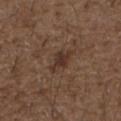<tbp_lesion>
  <biopsy_status>not biopsied; imaged during a skin examination</biopsy_status>
  <site>arm</site>
  <image>
    <source>total-body photography crop</source>
    <field_of_view_mm>15</field_of_view_mm>
  </image>
  <patient>
    <sex>male</sex>
    <age_approx>50</age_approx>
  </patient>
</tbp_lesion>